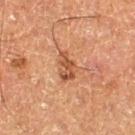Q: Is there a histopathology result?
A: imaged on a skin check; not biopsied
Q: What is the imaging modality?
A: 15 mm crop, total-body photography
Q: Where on the body is the lesion?
A: the left thigh
Q: What is the lesion's diameter?
A: ≈3.5 mm
Q: Patient demographics?
A: male, about 75 years old